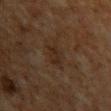Impression:
Part of a total-body skin-imaging series; this lesion was reviewed on a skin check and was not flagged for biopsy.